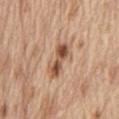notes — imaged on a skin check; not biopsied
lesion size — ~4.5 mm (longest diameter)
site — the abdomen
imaging modality — ~15 mm crop, total-body skin-cancer survey
subject — male, approximately 70 years of age
lighting — white-light